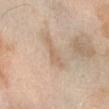Assessment: This lesion was catalogued during total-body skin photography and was not selected for biopsy. Image and clinical context: From the right forearm. Captured under cross-polarized illumination. A roughly 15 mm field-of-view crop from a total-body skin photograph. The subject is a male aged 53 to 57.